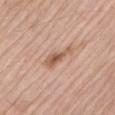Captured during whole-body skin photography for melanoma surveillance; the lesion was not biopsied.
A close-up tile cropped from a whole-body skin photograph, about 15 mm across.
Approximately 4 mm at its widest.
The lesion is located on the left upper arm.
A male subject roughly 75 years of age.
Captured under white-light illumination.